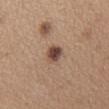Impression:
The lesion was photographed on a routine skin check and not biopsied; there is no pathology result.
Image and clinical context:
The lesion is on the upper back. Captured under white-light illumination. Automated tile analysis of the lesion measured an area of roughly 5 mm², a shape eccentricity near 0.6, and two-axis asymmetry of about 0.2. The software also gave an automated nevus-likeness rating near 90 out of 100 and a detector confidence of about 100 out of 100 that the crop contains a lesion. A female subject aged 38 to 42. A close-up tile cropped from a whole-body skin photograph, about 15 mm across. Measured at roughly 2.5 mm in maximum diameter.